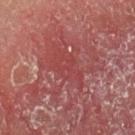{
  "biopsy_status": "not biopsied; imaged during a skin examination",
  "patient": {
    "sex": "male",
    "age_approx": 55
  },
  "site": "left upper arm",
  "image": {
    "source": "total-body photography crop",
    "field_of_view_mm": 15
  },
  "lighting": "cross-polarized"
}